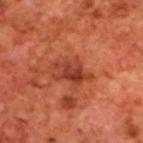Q: Was a biopsy performed?
A: no biopsy performed (imaged during a skin exam)
Q: What is the anatomic site?
A: the back
Q: What kind of image is this?
A: 15 mm crop, total-body photography
Q: Patient demographics?
A: male, in their 70s